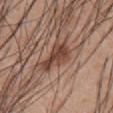Impression: The lesion was photographed on a routine skin check and not biopsied; there is no pathology result. Clinical summary: Automated tile analysis of the lesion measured a shape eccentricity near 0.85. And it measured an average lesion color of about L≈43 a*≈20 b*≈27 (CIELAB) and a normalized border contrast of about 9. It also reported a border-irregularity index near 5.5/10, a color-variation rating of about 3.5/10, and radial color variation of about 1. The lesion is on the front of the torso. A male subject approximately 55 years of age. A roughly 15 mm field-of-view crop from a total-body skin photograph.